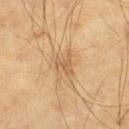follow-up — no biopsy performed (imaged during a skin exam) | lighting — cross-polarized | subject — male, aged 68–72 | location — the left thigh | image — total-body-photography crop, ~15 mm field of view | automated lesion analysis — a lesion area of about 5 mm², an eccentricity of roughly 0.7, and two-axis asymmetry of about 0.3; an average lesion color of about L≈57 a*≈18 b*≈36 (CIELAB); a border-irregularity index near 4/10, internal color variation of about 3.5 on a 0–10 scale, and a peripheral color-asymmetry measure near 1.5.